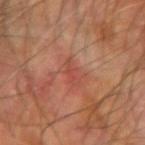- workup · catalogued during a skin exam; not biopsied
- diameter · ≈3 mm
- subject · male, aged approximately 70
- site · the left forearm
- acquisition · ~15 mm crop, total-body skin-cancer survey
- illumination · cross-polarized illumination